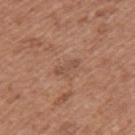Q: How large is the lesion?
A: ~3 mm (longest diameter)
Q: Automated lesion metrics?
A: a lesion area of about 2.5 mm², a shape eccentricity near 0.9, and two-axis asymmetry of about 0.25; an average lesion color of about L≈50 a*≈20 b*≈28 (CIELAB) and a lesion-to-skin contrast of about 5 (normalized; higher = more distinct); a nevus-likeness score of about 0/100 and lesion-presence confidence of about 100/100
Q: How was the tile lit?
A: white-light illumination
Q: Where on the body is the lesion?
A: the left upper arm
Q: Patient demographics?
A: female, approximately 50 years of age
Q: What is the imaging modality?
A: 15 mm crop, total-body photography